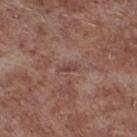| key | value |
|---|---|
| notes | imaged on a skin check; not biopsied |
| imaging modality | ~15 mm tile from a whole-body skin photo |
| patient | male, aged approximately 55 |
| anatomic site | the left lower leg |